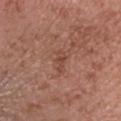Context: A close-up tile cropped from a whole-body skin photograph, about 15 mm across. Approximately 3 mm at its widest. This is a white-light tile. The lesion is on the head or neck. The patient is a female approximately 55 years of age.